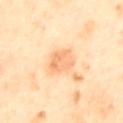notes = imaged on a skin check; not biopsied
patient = male, aged 63–67
body site = the back
automated lesion analysis = an automated nevus-likeness rating near 65 out of 100 and lesion-presence confidence of about 100/100
illumination = cross-polarized
image source = ~15 mm tile from a whole-body skin photo